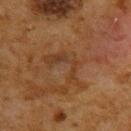workup — total-body-photography surveillance lesion; no biopsy
image — ~15 mm tile from a whole-body skin photo
illumination — cross-polarized illumination
automated metrics — lesion-presence confidence of about 100/100
location — the upper back
lesion diameter — about 6 mm
patient — male, aged 58 to 62